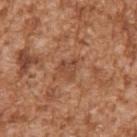Recorded during total-body skin imaging; not selected for excision or biopsy.
A male subject, in their mid- to late 40s.
Approximately 2.5 mm at its widest.
The lesion is located on the right upper arm.
This image is a 15 mm lesion crop taken from a total-body photograph.